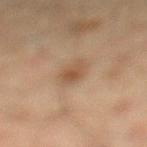Q: Was this lesion biopsied?
A: total-body-photography surveillance lesion; no biopsy
Q: Patient demographics?
A: male, aged approximately 45
Q: How large is the lesion?
A: ≈3 mm
Q: What kind of image is this?
A: ~15 mm tile from a whole-body skin photo
Q: Where on the body is the lesion?
A: the left lower leg
Q: Illumination type?
A: cross-polarized